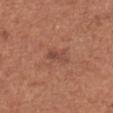biopsy status=catalogued during a skin exam; not biopsied | illumination=white-light | automated lesion analysis=a lesion area of about 3 mm², a shape eccentricity near 0.9, and a symmetry-axis asymmetry near 0.35; a lesion–skin lightness drop of about 8; border irregularity of about 3.5 on a 0–10 scale and internal color variation of about 0.5 on a 0–10 scale | image source=15 mm crop, total-body photography | body site=the chest | subject=female, aged around 50 | lesion diameter=about 2.5 mm.